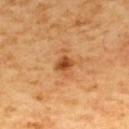imaging modality: 15 mm crop, total-body photography | patient: male, roughly 60 years of age | diameter: ~2 mm (longest diameter) | automated metrics: a lesion color around L≈49 a*≈27 b*≈41 in CIELAB and a normalized border contrast of about 9; a border-irregularity rating of about 2/10, internal color variation of about 2.5 on a 0–10 scale, and a peripheral color-asymmetry measure near 1 | site: the upper back | tile lighting: cross-polarized.